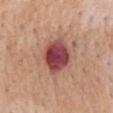Notes:
• biopsy status — catalogued during a skin exam; not biopsied
• location — the mid back
• patient — male, aged approximately 65
• lesion size — ≈4 mm
• illumination — white-light
• acquisition — total-body-photography crop, ~15 mm field of view